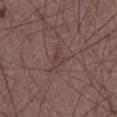Recorded during total-body skin imaging; not selected for excision or biopsy. On the abdomen. Captured under white-light illumination. Approximately 3 mm at its widest. A male subject about 60 years old. Cropped from a whole-body photographic skin survey; the tile spans about 15 mm. An algorithmic analysis of the crop reported an outline eccentricity of about 0.8 (0 = round, 1 = elongated) and two-axis asymmetry of about 0.45.